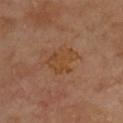notes = total-body-photography surveillance lesion; no biopsy
body site = the front of the torso
patient = male, about 70 years old
lighting = cross-polarized
acquisition = 15 mm crop, total-body photography
automated metrics = a lesion color around L≈43 a*≈20 b*≈34 in CIELAB and about 5 CIELAB-L* units darker than the surrounding skin
size = ~4 mm (longest diameter)